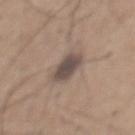  biopsy_status: not biopsied; imaged during a skin examination